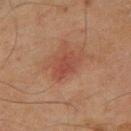{"automated_metrics": {"area_mm2_approx": 7.0, "shape_asymmetry": 0.25, "vs_skin_darker_L": 6.0, "vs_skin_contrast_norm": 5.5, "border_irregularity_0_10": 2.5, "color_variation_0_10": 3.0, "peripheral_color_asymmetry": 1.5, "nevus_likeness_0_100": 30, "lesion_detection_confidence_0_100": 100}, "image": {"source": "total-body photography crop", "field_of_view_mm": 15}, "site": "mid back", "patient": {"sex": "male", "age_approx": 45}}